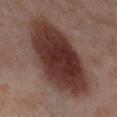Part of a total-body skin-imaging series; this lesion was reviewed on a skin check and was not flagged for biopsy. From the leg. The tile uses cross-polarized illumination. The subject is a female aged 53 to 57. This image is a 15 mm lesion crop taken from a total-body photograph. The total-body-photography lesion software estimated a mean CIELAB color near L≈35 a*≈19 b*≈22 and a lesion–skin lightness drop of about 15. The software also gave a border-irregularity rating of about 2/10, a color-variation rating of about 5.5/10, and radial color variation of about 1.5. The recorded lesion diameter is about 11.5 mm.